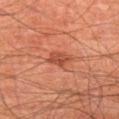{
  "biopsy_status": "not biopsied; imaged during a skin examination",
  "site": "left thigh",
  "image": {
    "source": "total-body photography crop",
    "field_of_view_mm": 15
  },
  "lighting": "cross-polarized",
  "patient": {
    "sex": "male",
    "age_approx": 65
  }
}